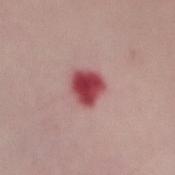Q: Was a biopsy performed?
A: imaged on a skin check; not biopsied
Q: What kind of image is this?
A: 15 mm crop, total-body photography
Q: How large is the lesion?
A: ~3.5 mm (longest diameter)
Q: Who is the patient?
A: female, roughly 50 years of age
Q: Where on the body is the lesion?
A: the chest
Q: Illumination type?
A: white-light